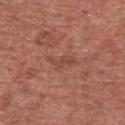The lesion was photographed on a routine skin check and not biopsied; there is no pathology result. Imaged with white-light lighting. The subject is a male aged 73–77. A region of skin cropped from a whole-body photographic capture, roughly 15 mm wide. About 4 mm across. Located on the chest.